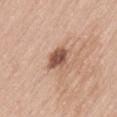Q: Where on the body is the lesion?
A: the lower back
Q: What are the patient's age and sex?
A: female, aged 43 to 47
Q: How was this image acquired?
A: 15 mm crop, total-body photography
Q: Illumination type?
A: white-light illumination